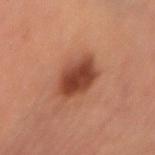Imaged during a routine full-body skin examination; the lesion was not biopsied and no histopathology is available.
A 15 mm crop from a total-body photograph taken for skin-cancer surveillance.
Located on the leg.
A female patient, in their mid- to late 60s.
Imaged with cross-polarized lighting.
Longest diameter approximately 5 mm.